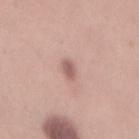Case summary:
– notes · catalogued during a skin exam; not biopsied